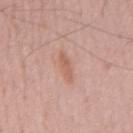{"biopsy_status": "not biopsied; imaged during a skin examination", "lesion_size": {"long_diameter_mm_approx": 3.5}, "image": {"source": "total-body photography crop", "field_of_view_mm": 15}, "patient": {"sex": "male", "age_approx": 55}, "lighting": "white-light"}